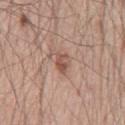<case>
  <biopsy_status>not biopsied; imaged during a skin examination</biopsy_status>
  <patient>
    <sex>male</sex>
    <age_approx>55</age_approx>
  </patient>
  <image>
    <source>total-body photography crop</source>
    <field_of_view_mm>15</field_of_view_mm>
  </image>
  <site>chest</site>
</case>